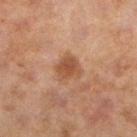<case>
  <biopsy_status>not biopsied; imaged during a skin examination</biopsy_status>
  <patient>
    <sex>female</sex>
    <age_approx>60</age_approx>
  </patient>
  <image>
    <source>total-body photography crop</source>
    <field_of_view_mm>15</field_of_view_mm>
  </image>
  <lighting>cross-polarized</lighting>
  <lesion_size>
    <long_diameter_mm_approx>3.0</long_diameter_mm_approx>
  </lesion_size>
  <site>leg</site>
  <automated_metrics>
    <area_mm2_approx>6.5</area_mm2_approx>
    <eccentricity>0.45</eccentricity>
    <shape_asymmetry>0.25</shape_asymmetry>
  </automated_metrics>
</case>